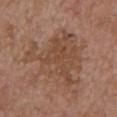– workup · total-body-photography surveillance lesion; no biopsy
– anatomic site · the chest
– lighting · white-light
– automated metrics · an average lesion color of about L≈47 a*≈19 b*≈29 (CIELAB), about 7 CIELAB-L* units darker than the surrounding skin, and a normalized border contrast of about 5.5; a border-irregularity rating of about 8.5/10, a within-lesion color-variation index near 3.5/10, and a peripheral color-asymmetry measure near 1.5; a nevus-likeness score of about 0/100 and a lesion-detection confidence of about 100/100
– diameter · ≈8.5 mm
– patient · female, aged 63–67
– imaging modality · ~15 mm crop, total-body skin-cancer survey